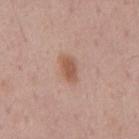Captured during whole-body skin photography for melanoma surveillance; the lesion was not biopsied. Automated tile analysis of the lesion measured a border-irregularity index near 1.5/10 and a peripheral color-asymmetry measure near 1. The lesion is on the mid back. The subject is a male about 45 years old. This is a white-light tile. Cropped from a whole-body photographic skin survey; the tile spans about 15 mm. The recorded lesion diameter is about 3.5 mm.